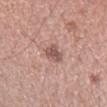Clinical impression: Part of a total-body skin-imaging series; this lesion was reviewed on a skin check and was not flagged for biopsy. Acquisition and patient details: The lesion is located on the right forearm. Measured at roughly 2.5 mm in maximum diameter. A roughly 15 mm field-of-view crop from a total-body skin photograph. Imaged with white-light lighting. A female subject, about 35 years old.